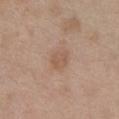This lesion was catalogued during total-body skin photography and was not selected for biopsy. Cropped from a total-body skin-imaging series; the visible field is about 15 mm. Imaged with white-light lighting. Automated tile analysis of the lesion measured a lesion area of about 5 mm². And it measured a lesion–skin lightness drop of about 6. And it measured a classifier nevus-likeness of about 5/100. A female subject, roughly 40 years of age. The lesion is on the front of the torso. The lesion's longest dimension is about 3 mm.